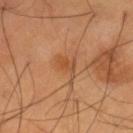Recorded during total-body skin imaging; not selected for excision or biopsy. The lesion's longest dimension is about 3.5 mm. Automated image analysis of the tile measured border irregularity of about 7 on a 0–10 scale, a within-lesion color-variation index near 2.5/10, and radial color variation of about 1. And it measured a nevus-likeness score of about 20/100 and a lesion-detection confidence of about 100/100. Captured under cross-polarized illumination. The patient is a male approximately 55 years of age. The lesion is located on the leg. A 15 mm close-up tile from a total-body photography series done for melanoma screening.